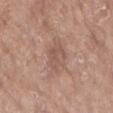Impression: The lesion was photographed on a routine skin check and not biopsied; there is no pathology result. Context: A 15 mm close-up extracted from a 3D total-body photography capture. Located on the left forearm. Measured at roughly 3.5 mm in maximum diameter. The tile uses white-light illumination. A female patient, in their mid- to late 70s.